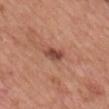Q: Automated lesion metrics?
A: an area of roughly 4.5 mm², a shape eccentricity near 0.7, and two-axis asymmetry of about 0.25; border irregularity of about 2.5 on a 0–10 scale, a within-lesion color-variation index near 4/10, and radial color variation of about 1.5; a classifier nevus-likeness of about 70/100
Q: Where on the body is the lesion?
A: the chest
Q: Patient demographics?
A: male, in their mid- to late 50s
Q: Illumination type?
A: white-light
Q: How large is the lesion?
A: ~3 mm (longest diameter)
Q: How was this image acquired?
A: total-body-photography crop, ~15 mm field of view